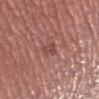- lesion size: ≈3 mm
- automated metrics: a lesion area of about 3 mm², a shape eccentricity near 0.85, and a symmetry-axis asymmetry near 0.4; an automated nevus-likeness rating near 0 out of 100 and a detector confidence of about 95 out of 100 that the crop contains a lesion
- location: the left lower leg
- patient: female, in their 40s
- tile lighting: white-light illumination
- image: 15 mm crop, total-body photography